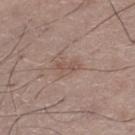Context: A 15 mm close-up extracted from a 3D total-body photography capture. The patient is a male roughly 50 years of age. From the leg.